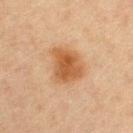Imaged during a routine full-body skin examination; the lesion was not biopsied and no histopathology is available. A female subject, about 45 years old. Located on the left upper arm. About 4 mm across. This image is a 15 mm lesion crop taken from a total-body photograph.